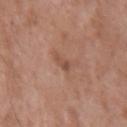Clinical impression:
Imaged during a routine full-body skin examination; the lesion was not biopsied and no histopathology is available.
Image and clinical context:
A 15 mm close-up extracted from a 3D total-body photography capture. On the left upper arm. The subject is a male aged 48–52.